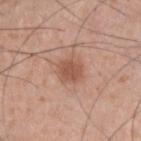imaging modality: ~15 mm tile from a whole-body skin photo
automated metrics: a lesion area of about 6 mm²; a mean CIELAB color near L≈53 a*≈23 b*≈30; a border-irregularity rating of about 2/10, a within-lesion color-variation index near 2/10, and peripheral color asymmetry of about 1; an automated nevus-likeness rating near 85 out of 100 and lesion-presence confidence of about 100/100
location: the upper back
subject: male, aged around 35
tile lighting: white-light illumination